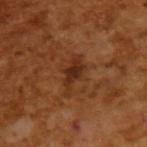biopsy status: catalogued during a skin exam; not biopsied
lesion diameter: ~4.5 mm (longest diameter)
tile lighting: cross-polarized illumination
imaging modality: ~15 mm tile from a whole-body skin photo
patient: male, approximately 65 years of age